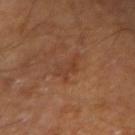{"biopsy_status": "not biopsied; imaged during a skin examination", "patient": {"sex": "male", "age_approx": 70}, "lighting": "cross-polarized", "site": "arm", "automated_metrics": {"eccentricity": 0.75, "shape_asymmetry": 0.4, "cielab_L": 39, "cielab_a": 21, "cielab_b": 31}, "lesion_size": {"long_diameter_mm_approx": 3.5}, "image": {"source": "total-body photography crop", "field_of_view_mm": 15}}